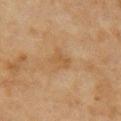workup: total-body-photography surveillance lesion; no biopsy | image: ~15 mm tile from a whole-body skin photo | subject: female, aged around 60 | illumination: cross-polarized illumination | TBP lesion metrics: a footprint of about 5 mm² and a symmetry-axis asymmetry near 0.25; a mean CIELAB color near L≈51 a*≈17 b*≈35 and a lesion-to-skin contrast of about 5 (normalized; higher = more distinct); radial color variation of about 0.5; an automated nevus-likeness rating near 0 out of 100 and lesion-presence confidence of about 100/100 | size: ≈3 mm | site: the right upper arm.